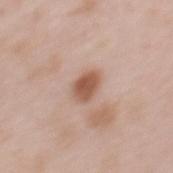Clinical impression:
The lesion was photographed on a routine skin check and not biopsied; there is no pathology result.